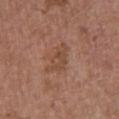This lesion was catalogued during total-body skin photography and was not selected for biopsy. A 15 mm close-up extracted from a 3D total-body photography capture. Automated tile analysis of the lesion measured a lesion color around L≈47 a*≈20 b*≈30 in CIELAB, a lesion–skin lightness drop of about 7, and a lesion-to-skin contrast of about 6 (normalized; higher = more distinct). The analysis additionally found an automated nevus-likeness rating near 0 out of 100 and lesion-presence confidence of about 100/100. The lesion is on the front of the torso. A female patient in their mid-60s. The recorded lesion diameter is about 4.5 mm. This is a white-light tile.